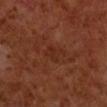<lesion>
  <biopsy_status>not biopsied; imaged during a skin examination</biopsy_status>
  <site>right forearm</site>
  <lesion_size>
    <long_diameter_mm_approx>2.5</long_diameter_mm_approx>
  </lesion_size>
  <lighting>cross-polarized</lighting>
  <patient>
    <sex>male</sex>
    <age_approx>60</age_approx>
  </patient>
  <image>
    <source>total-body photography crop</source>
    <field_of_view_mm>15</field_of_view_mm>
  </image>
</lesion>